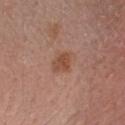Clinical impression:
No biopsy was performed on this lesion — it was imaged during a full skin examination and was not determined to be concerning.
Acquisition and patient details:
Longest diameter approximately 3 mm. The subject is a female aged 48 to 52. Captured under white-light illumination. Located on the front of the torso. A roughly 15 mm field-of-view crop from a total-body skin photograph. Automated image analysis of the tile measured a mean CIELAB color near L≈48 a*≈23 b*≈30, roughly 9 lightness units darker than nearby skin, and a lesion-to-skin contrast of about 7 (normalized; higher = more distinct). It also reported an automated nevus-likeness rating near 70 out of 100 and lesion-presence confidence of about 100/100.